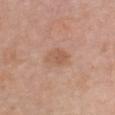Captured during whole-body skin photography for melanoma surveillance; the lesion was not biopsied.
The subject is a female roughly 60 years of age.
Cropped from a total-body skin-imaging series; the visible field is about 15 mm.
The lesion is located on the chest.